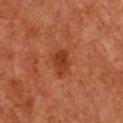This lesion was catalogued during total-body skin photography and was not selected for biopsy.